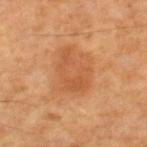- notes — imaged on a skin check; not biopsied